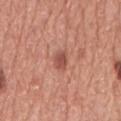Clinical impression:
This lesion was catalogued during total-body skin photography and was not selected for biopsy.
Background:
The patient is a male approximately 75 years of age. On the mid back. This image is a 15 mm lesion crop taken from a total-body photograph. Longest diameter approximately 2.5 mm.